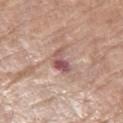biopsy status — imaged on a skin check; not biopsied
acquisition — ~15 mm crop, total-body skin-cancer survey
TBP lesion metrics — a classifier nevus-likeness of about 0/100
patient — female, in their mid- to late 70s
anatomic site — the right thigh
tile lighting — white-light illumination
lesion diameter — ~3.5 mm (longest diameter)